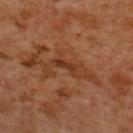The lesion was tiled from a total-body skin photograph and was not biopsied. From the upper back. A 15 mm crop from a total-body photograph taken for skin-cancer surveillance. The subject is a female aged around 55.